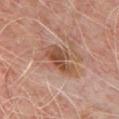Q: Automated lesion metrics?
A: an eccentricity of roughly 0.85 and a symmetry-axis asymmetry near 0.25; a mean CIELAB color near L≈49 a*≈21 b*≈30, a lesion–skin lightness drop of about 11, and a normalized border contrast of about 8.5; border irregularity of about 3.5 on a 0–10 scale and radial color variation of about 1.5
Q: What is the imaging modality?
A: ~15 mm tile from a whole-body skin photo
Q: How was the tile lit?
A: cross-polarized illumination
Q: Patient demographics?
A: male, in their 50s
Q: Lesion size?
A: ≈5 mm
Q: What is the anatomic site?
A: the front of the torso